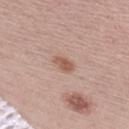The lesion was photographed on a routine skin check and not biopsied; there is no pathology result.